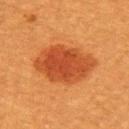Assessment: No biopsy was performed on this lesion — it was imaged during a full skin examination and was not determined to be concerning. Acquisition and patient details: A female subject aged around 55. A roughly 15 mm field-of-view crop from a total-body skin photograph. The tile uses cross-polarized illumination. On the back. Longest diameter approximately 7.5 mm.